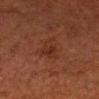<tbp_lesion>
<biopsy_status>not biopsied; imaged during a skin examination</biopsy_status>
<site>head or neck</site>
<patient>
  <sex>male</sex>
  <age_approx>65</age_approx>
</patient>
<image>
  <source>total-body photography crop</source>
  <field_of_view_mm>15</field_of_view_mm>
</image>
<lighting>cross-polarized</lighting>
<automated_metrics>
  <area_mm2_approx>6.5</area_mm2_approx>
  <eccentricity>0.7</eccentricity>
  <shape_asymmetry>0.45</shape_asymmetry>
</automated_metrics>
<lesion_size>
  <long_diameter_mm_approx>3.5</long_diameter_mm_approx>
</lesion_size>
</tbp_lesion>